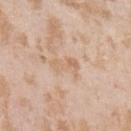Q: Was a biopsy performed?
A: imaged on a skin check; not biopsied
Q: What is the lesion's diameter?
A: about 3.5 mm
Q: Patient demographics?
A: female, approximately 25 years of age
Q: What is the imaging modality?
A: 15 mm crop, total-body photography
Q: What lighting was used for the tile?
A: white-light
Q: Where on the body is the lesion?
A: the left upper arm
Q: Automated lesion metrics?
A: a footprint of about 5.5 mm², a shape eccentricity near 0.85, and a shape-asymmetry score of about 0.3 (0 = symmetric); an average lesion color of about L≈66 a*≈17 b*≈32 (CIELAB) and roughly 6 lightness units darker than nearby skin; a nevus-likeness score of about 0/100 and lesion-presence confidence of about 100/100